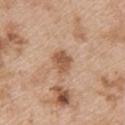Clinical impression: Recorded during total-body skin imaging; not selected for excision or biopsy. Acquisition and patient details: Located on the upper back. Imaged with white-light lighting. Automated image analysis of the tile measured a lesion area of about 5.5 mm², a shape eccentricity near 0.55, and two-axis asymmetry of about 0.15. The analysis additionally found a border-irregularity rating of about 1.5/10, internal color variation of about 2.5 on a 0–10 scale, and a peripheral color-asymmetry measure near 1. A female subject, approximately 45 years of age. A 15 mm close-up tile from a total-body photography series done for melanoma screening.